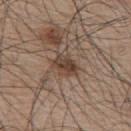Findings:
- biopsy status — total-body-photography surveillance lesion; no biopsy
- anatomic site — the mid back
- image — ~15 mm tile from a whole-body skin photo
- subject — male, aged 73 to 77
- illumination — white-light
- size — ~3.5 mm (longest diameter)